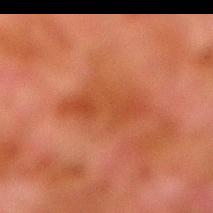Recorded during total-body skin imaging; not selected for excision or biopsy.
A male patient, approximately 80 years of age.
A 15 mm crop from a total-body photograph taken for skin-cancer surveillance.
On the right lower leg.
This is a cross-polarized tile.
The total-body-photography lesion software estimated a lesion area of about 12 mm² and a shape-asymmetry score of about 0.3 (0 = symmetric). The analysis additionally found a lesion color around L≈38 a*≈26 b*≈33 in CIELAB, about 6 CIELAB-L* units darker than the surrounding skin, and a lesion-to-skin contrast of about 6 (normalized; higher = more distinct).
About 6 mm across.